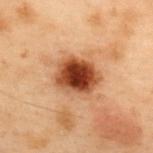Acquisition and patient details:
The lesion is on the upper back. A 15 mm close-up extracted from a 3D total-body photography capture. The subject is a male in their mid-50s. This is a cross-polarized tile. About 4.5 mm across.